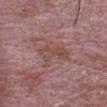Findings:
– follow-up · total-body-photography surveillance lesion; no biopsy
– body site · the head or neck
– image · 15 mm crop, total-body photography
– subject · male, aged around 65
– illumination · white-light illumination
– automated lesion analysis · a footprint of about 7.5 mm², a shape eccentricity near 0.85, and a symmetry-axis asymmetry near 0.35; an average lesion color of about L≈47 a*≈21 b*≈22 (CIELAB), about 7 CIELAB-L* units darker than the surrounding skin, and a lesion-to-skin contrast of about 6 (normalized; higher = more distinct); border irregularity of about 4 on a 0–10 scale and a peripheral color-asymmetry measure near 1
– size · ~4 mm (longest diameter)